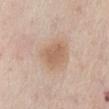On the front of the torso.
The subject is a male aged 73–77.
This image is a 15 mm lesion crop taken from a total-body photograph.